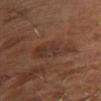Captured during whole-body skin photography for melanoma surveillance; the lesion was not biopsied. About 5.5 mm across. The total-body-photography lesion software estimated an automated nevus-likeness rating near 0 out of 100 and lesion-presence confidence of about 100/100. A 15 mm close-up extracted from a 3D total-body photography capture. The subject is a male in their mid-60s. Captured under cross-polarized illumination.